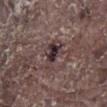Recorded during total-body skin imaging; not selected for excision or biopsy. The lesion is located on the right lower leg. Automated image analysis of the tile measured an area of roughly 7.5 mm², an outline eccentricity of about 0.75 (0 = round, 1 = elongated), and a shape-asymmetry score of about 0.4 (0 = symmetric). It also reported an average lesion color of about L≈34 a*≈16 b*≈13 (CIELAB) and a normalized border contrast of about 10.5. It also reported a border-irregularity index near 4.5/10, internal color variation of about 7.5 on a 0–10 scale, and radial color variation of about 2.5. A roughly 15 mm field-of-view crop from a total-body skin photograph. A male patient approximately 80 years of age. Imaged with white-light lighting.